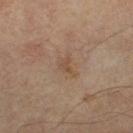Findings:
* notes: total-body-photography surveillance lesion; no biopsy
* subject: male, aged approximately 65
* body site: the right thigh
* illumination: cross-polarized
* imaging modality: 15 mm crop, total-body photography
* diameter: ≈2.5 mm